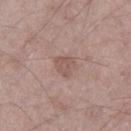follow-up: no biopsy performed (imaged during a skin exam)
image-analysis metrics: an average lesion color of about L≈54 a*≈19 b*≈23 (CIELAB), a lesion–skin lightness drop of about 7, and a lesion-to-skin contrast of about 5.5 (normalized; higher = more distinct); a color-variation rating of about 2/10
anatomic site: the leg
image: ~15 mm crop, total-body skin-cancer survey
diameter: ≈3 mm
patient: male, aged approximately 50